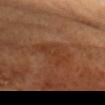Q: How was the tile lit?
A: cross-polarized
Q: Patient demographics?
A: male, aged 58 to 62
Q: How large is the lesion?
A: ≈4 mm
Q: What is the imaging modality?
A: ~15 mm tile from a whole-body skin photo
Q: What is the anatomic site?
A: the head or neck
Q: What did automated image analysis measure?
A: a footprint of about 7 mm² and two-axis asymmetry of about 0.45; an average lesion color of about L≈35 a*≈22 b*≈31 (CIELAB) and a lesion–skin lightness drop of about 5; a border-irregularity index near 6/10, a within-lesion color-variation index near 2/10, and peripheral color asymmetry of about 1; a classifier nevus-likeness of about 0/100 and a detector confidence of about 100 out of 100 that the crop contains a lesion